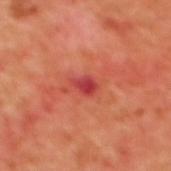Q: Was this lesion biopsied?
A: total-body-photography surveillance lesion; no biopsy
Q: Lesion location?
A: the upper back
Q: Automated lesion metrics?
A: a classifier nevus-likeness of about 0/100 and a detector confidence of about 100 out of 100 that the crop contains a lesion
Q: How was the tile lit?
A: cross-polarized
Q: What is the imaging modality?
A: ~15 mm crop, total-body skin-cancer survey
Q: Lesion size?
A: about 3 mm
Q: Patient demographics?
A: female, approximately 40 years of age